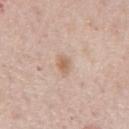<lesion>
  <biopsy_status>not biopsied; imaged during a skin examination</biopsy_status>
  <patient>
    <sex>male</sex>
    <age_approx>75</age_approx>
  </patient>
  <lighting>white-light</lighting>
  <site>mid back</site>
  <lesion_size>
    <long_diameter_mm_approx>2.5</long_diameter_mm_approx>
  </lesion_size>
  <image>
    <source>total-body photography crop</source>
    <field_of_view_mm>15</field_of_view_mm>
  </image>
  <automated_metrics>
    <cielab_L>63</cielab_L>
    <cielab_a>17</cielab_a>
    <cielab_b>30</cielab_b>
    <vs_skin_contrast_norm>6.5</vs_skin_contrast_norm>
    <border_irregularity_0_10>2.5</border_irregularity_0_10>
    <color_variation_0_10>3.0</color_variation_0_10>
    <peripheral_color_asymmetry>1.0</peripheral_color_asymmetry>
  </automated_metrics>
</lesion>